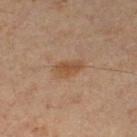– notes · total-body-photography surveillance lesion; no biopsy
– body site · the left lower leg
– image source · ~15 mm tile from a whole-body skin photo
– tile lighting · cross-polarized
– image-analysis metrics · a mean CIELAB color near L≈45 a*≈18 b*≈31, a lesion–skin lightness drop of about 8, and a normalized border contrast of about 7.5; a classifier nevus-likeness of about 85/100 and a lesion-detection confidence of about 100/100
– lesion diameter · ~3 mm (longest diameter)
– patient · male, aged 58 to 62